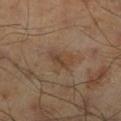Q: Was this lesion biopsied?
A: catalogued during a skin exam; not biopsied
Q: What kind of image is this?
A: total-body-photography crop, ~15 mm field of view
Q: Who is the patient?
A: male, aged approximately 45
Q: Lesion size?
A: ≈3 mm
Q: Lesion location?
A: the right lower leg
Q: What lighting was used for the tile?
A: cross-polarized